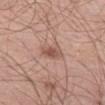Captured during whole-body skin photography for melanoma surveillance; the lesion was not biopsied.
The lesion is on the left thigh.
A male subject in their 70s.
A close-up tile cropped from a whole-body skin photograph, about 15 mm across.